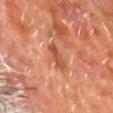Q: Was a biopsy performed?
A: catalogued during a skin exam; not biopsied
Q: What is the anatomic site?
A: the upper back
Q: What kind of image is this?
A: ~15 mm crop, total-body skin-cancer survey
Q: How was the tile lit?
A: cross-polarized illumination
Q: What are the patient's age and sex?
A: male, aged approximately 60